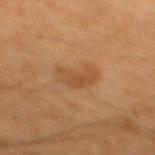Notes:
* workup — no biopsy performed (imaged during a skin exam)
* imaging modality — total-body-photography crop, ~15 mm field of view
* site — the mid back
* subject — male, approximately 65 years of age
* diameter — ~4 mm (longest diameter)
* illumination — cross-polarized illumination